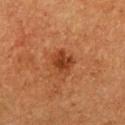Assessment: Captured during whole-body skin photography for melanoma surveillance; the lesion was not biopsied. Image and clinical context: The lesion's longest dimension is about 3 mm. A male subject about 75 years old. Cropped from a total-body skin-imaging series; the visible field is about 15 mm. Located on the left upper arm.